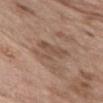Assessment: Part of a total-body skin-imaging series; this lesion was reviewed on a skin check and was not flagged for biopsy. Clinical summary: A region of skin cropped from a whole-body photographic capture, roughly 15 mm wide. A female subject aged approximately 75. The lesion's longest dimension is about 4 mm. Automated tile analysis of the lesion measured a mean CIELAB color near L≈50 a*≈17 b*≈27, a lesion–skin lightness drop of about 7, and a normalized border contrast of about 5.5. It also reported a border-irregularity rating of about 6.5/10, a color-variation rating of about 2/10, and peripheral color asymmetry of about 1. Located on the front of the torso. This is a white-light tile.